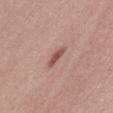lighting = white-light illumination; diameter = ≈3 mm; anatomic site = the abdomen; imaging modality = ~15 mm tile from a whole-body skin photo; patient = female, aged 23 to 27.